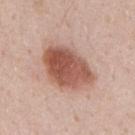biopsy_status: not biopsied; imaged during a skin examination
image:
  source: total-body photography crop
  field_of_view_mm: 15
patient:
  sex: male
  age_approx: 40
site: mid back